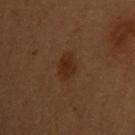Captured during whole-body skin photography for melanoma surveillance; the lesion was not biopsied.